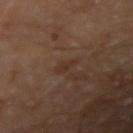Clinical impression: Imaged during a routine full-body skin examination; the lesion was not biopsied and no histopathology is available. Clinical summary: A male patient, roughly 60 years of age. Approximately 2.5 mm at its widest. The lesion is located on the right upper arm. Cropped from a total-body skin-imaging series; the visible field is about 15 mm.